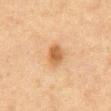biopsy status: total-body-photography surveillance lesion; no biopsy
body site: the abdomen
illumination: cross-polarized illumination
lesion diameter: ~3 mm (longest diameter)
subject: male, aged around 75
acquisition: 15 mm crop, total-body photography